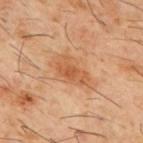Q: What is the lesion's diameter?
A: ~5.5 mm (longest diameter)
Q: Lesion location?
A: the upper back
Q: What is the imaging modality?
A: 15 mm crop, total-body photography
Q: What are the patient's age and sex?
A: male, approximately 60 years of age